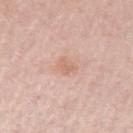<record>
<biopsy_status>not biopsied; imaged during a skin examination</biopsy_status>
<image>
  <source>total-body photography crop</source>
  <field_of_view_mm>15</field_of_view_mm>
</image>
<site>left upper arm</site>
<lighting>white-light</lighting>
<automated_metrics>
  <area_mm2_approx>4.0</area_mm2_approx>
  <eccentricity>0.7</eccentricity>
  <shape_asymmetry>0.3</shape_asymmetry>
  <cielab_L>66</cielab_L>
  <cielab_a>21</cielab_a>
  <cielab_b>29</cielab_b>
  <vs_skin_darker_L>7.0</vs_skin_darker_L>
</automated_metrics>
<patient>
  <sex>male</sex>
  <age_approx>65</age_approx>
</patient>
</record>